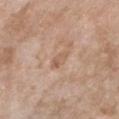workup: no biopsy performed (imaged during a skin exam)
patient: female, in their mid-70s
imaging modality: total-body-photography crop, ~15 mm field of view
anatomic site: the chest
size: about 3 mm
lighting: white-light
image-analysis metrics: a lesion area of about 3.5 mm², a shape eccentricity near 0.9, and two-axis asymmetry of about 0.2